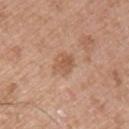No biopsy was performed on this lesion — it was imaged during a full skin examination and was not determined to be concerning.
From the left upper arm.
A 15 mm close-up extracted from a 3D total-body photography capture.
Captured under white-light illumination.
The patient is a male aged approximately 65.
Longest diameter approximately 3 mm.
Automated tile analysis of the lesion measured a lesion area of about 5.5 mm², a shape eccentricity near 0.65, and two-axis asymmetry of about 0.2. The software also gave a lesion color around L≈56 a*≈21 b*≈32 in CIELAB, roughly 8 lightness units darker than nearby skin, and a lesion-to-skin contrast of about 6 (normalized; higher = more distinct). The software also gave a border-irregularity index near 2/10, internal color variation of about 3 on a 0–10 scale, and a peripheral color-asymmetry measure near 1. It also reported an automated nevus-likeness rating near 0 out of 100 and lesion-presence confidence of about 100/100.